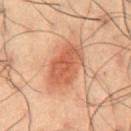Findings:
* biopsy status — total-body-photography surveillance lesion; no biopsy
* body site — the abdomen
* image-analysis metrics — an area of roughly 16 mm², a shape eccentricity near 0.75, and a shape-asymmetry score of about 0.2 (0 = symmetric); border irregularity of about 2 on a 0–10 scale, a color-variation rating of about 3.5/10, and a peripheral color-asymmetry measure near 1
* illumination — cross-polarized illumination
* patient — male, aged approximately 50
* image source — 15 mm crop, total-body photography
* size — ~5.5 mm (longest diameter)